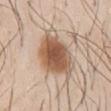  biopsy_status: not biopsied; imaged during a skin examination
  image:
    source: total-body photography crop
    field_of_view_mm: 15
  patient:
    sex: male
    age_approx: 30
  lighting: white-light
  site: abdomen
  automated_metrics:
    shape_asymmetry: 0.3
    vs_skin_darker_L: 15.0
    nevus_likeness_0_100: 95
    lesion_detection_confidence_0_100: 100
  lesion_size:
    long_diameter_mm_approx: 7.0